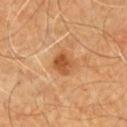The lesion was tiled from a total-body skin photograph and was not biopsied.
A region of skin cropped from a whole-body photographic capture, roughly 15 mm wide.
The tile uses cross-polarized illumination.
The lesion is located on the front of the torso.
The subject is a male roughly 60 years of age.